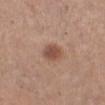A roughly 15 mm field-of-view crop from a total-body skin photograph. Measured at roughly 3 mm in maximum diameter. The subject is a female aged around 30. From the left lower leg. Captured under white-light illumination.